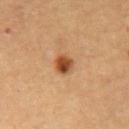Clinical impression: The lesion was tiled from a total-body skin photograph and was not biopsied. Background: Captured under cross-polarized illumination. The patient is a male aged approximately 65. The lesion-visualizer software estimated a lesion area of about 4.5 mm² and a symmetry-axis asymmetry near 0.2. The software also gave a lesion color around L≈45 a*≈22 b*≈36 in CIELAB, roughly 14 lightness units darker than nearby skin, and a normalized border contrast of about 10.5. It also reported a border-irregularity index near 1.5/10, internal color variation of about 5.5 on a 0–10 scale, and a peripheral color-asymmetry measure near 1.5. The software also gave an automated nevus-likeness rating near 95 out of 100 and a lesion-detection confidence of about 100/100. A 15 mm close-up extracted from a 3D total-body photography capture. On the upper back.